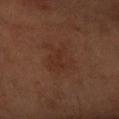biopsy_status: not biopsied; imaged during a skin examination
lesion_size:
  long_diameter_mm_approx: 4.5
image:
  source: total-body photography crop
  field_of_view_mm: 15
lighting: cross-polarized
patient:
  sex: male
  age_approx: 60
site: right forearm